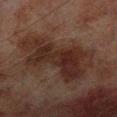Clinical summary: The lesion is on the right lower leg. A 15 mm close-up tile from a total-body photography series done for melanoma screening. A male subject aged approximately 70. The lesion's longest dimension is about 10 mm. Imaged with cross-polarized lighting.